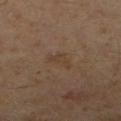Impression:
The lesion was tiled from a total-body skin photograph and was not biopsied.
Context:
Cropped from a whole-body photographic skin survey; the tile spans about 15 mm. From the right lower leg. The tile uses cross-polarized illumination. Measured at roughly 3 mm in maximum diameter. A male patient, approximately 60 years of age.